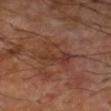Clinical impression: Captured during whole-body skin photography for melanoma surveillance; the lesion was not biopsied. Background: The tile uses cross-polarized illumination. About 5 mm across. The lesion is on the arm. A close-up tile cropped from a whole-body skin photograph, about 15 mm across. The patient is a male about 70 years old. Automated image analysis of the tile measured an average lesion color of about L≈35 a*≈21 b*≈27 (CIELAB) and a normalized lesion–skin contrast near 5.5.